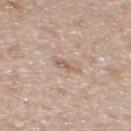| key | value |
|---|---|
| lighting | white-light illumination |
| image source | total-body-photography crop, ~15 mm field of view |
| lesion diameter | ≈2.5 mm |
| location | the upper back |
| subject | female, aged 28 to 32 |